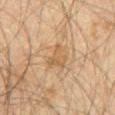Recorded during total-body skin imaging; not selected for excision or biopsy. Located on the abdomen. Automated image analysis of the tile measured an area of roughly 5 mm² and two-axis asymmetry of about 0.55. It also reported a border-irregularity index near 5.5/10, a color-variation rating of about 2.5/10, and radial color variation of about 0.5. The software also gave a classifier nevus-likeness of about 0/100 and a lesion-detection confidence of about 100/100. This image is a 15 mm lesion crop taken from a total-body photograph. Measured at roughly 3 mm in maximum diameter. A male subject, aged 58 to 62.